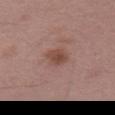The lesion was photographed on a routine skin check and not biopsied; there is no pathology result. A roughly 15 mm field-of-view crop from a total-body skin photograph. The subject is a female aged 48–52. From the leg.